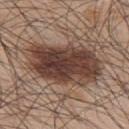biopsy status = no biopsy performed (imaged during a skin exam); acquisition = total-body-photography crop, ~15 mm field of view; diameter = ~8.5 mm (longest diameter); illumination = white-light illumination; patient = male, roughly 45 years of age; body site = the upper back.